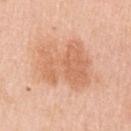Imaged during a routine full-body skin examination; the lesion was not biopsied and no histopathology is available.
The subject is a female in their mid-40s.
From the left upper arm.
A roughly 15 mm field-of-view crop from a total-body skin photograph.
The tile uses white-light illumination.
The lesion's longest dimension is about 7 mm.